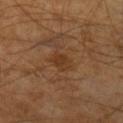workup: imaged on a skin check; not biopsied
image source: ~15 mm crop, total-body skin-cancer survey
illumination: cross-polarized
lesion size: about 2.5 mm
TBP lesion metrics: a lesion color around L≈35 a*≈20 b*≈32 in CIELAB and roughly 6 lightness units darker than nearby skin; an automated nevus-likeness rating near 10 out of 100
patient: male, aged 63 to 67